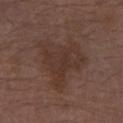Impression:
Imaged during a routine full-body skin examination; the lesion was not biopsied and no histopathology is available.
Clinical summary:
On the left forearm. A male patient, aged around 70. A lesion tile, about 15 mm wide, cut from a 3D total-body photograph.